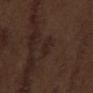Q: Is there a histopathology result?
A: no biopsy performed (imaged during a skin exam)
Q: How was this image acquired?
A: ~15 mm tile from a whole-body skin photo
Q: What is the anatomic site?
A: the mid back
Q: What are the patient's age and sex?
A: male, aged around 70